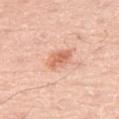The lesion was tiled from a total-body skin photograph and was not biopsied. On the left thigh. A male subject roughly 70 years of age. A lesion tile, about 15 mm wide, cut from a 3D total-body photograph. An algorithmic analysis of the crop reported a footprint of about 6 mm², a shape eccentricity near 0.7, and a symmetry-axis asymmetry near 0.2. And it measured border irregularity of about 2 on a 0–10 scale, a within-lesion color-variation index near 3/10, and radial color variation of about 1. This is a white-light tile.